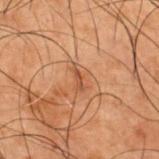Captured during whole-body skin photography for melanoma surveillance; the lesion was not biopsied. From the upper back. The patient is a male aged 48 to 52. A 15 mm close-up tile from a total-body photography series done for melanoma screening. The lesion's longest dimension is about 3 mm.